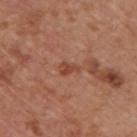Case summary:
• tile lighting — white-light
• lesion size — about 2.5 mm
• subject — male, about 65 years old
• image — total-body-photography crop, ~15 mm field of view
• location — the upper back